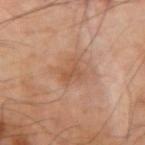The lesion was photographed on a routine skin check and not biopsied; there is no pathology result. About 3 mm across. This image is a 15 mm lesion crop taken from a total-body photograph. Located on the left arm. Automated tile analysis of the lesion measured a lesion color around L≈53 a*≈21 b*≈32 in CIELAB, about 7 CIELAB-L* units darker than the surrounding skin, and a normalized lesion–skin contrast near 5.5. It also reported a border-irregularity index near 5/10, a color-variation rating of about 3/10, and a peripheral color-asymmetry measure near 1. The patient is a male roughly 50 years of age.